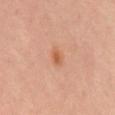  lesion_size:
    long_diameter_mm_approx: 2.5
  image:
    source: total-body photography crop
    field_of_view_mm: 15
  site: mid back
  patient:
    sex: male
    age_approx: 65